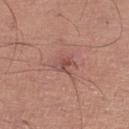{
  "patient": {
    "sex": "male",
    "age_approx": 55
  },
  "image": {
    "source": "total-body photography crop",
    "field_of_view_mm": 15
  },
  "lesion_size": {
    "long_diameter_mm_approx": 2.5
  },
  "lighting": "white-light",
  "site": "right lower leg"
}